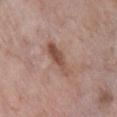Clinical impression:
Part of a total-body skin-imaging series; this lesion was reviewed on a skin check and was not flagged for biopsy.
Context:
Longest diameter approximately 4.5 mm. The lesion-visualizer software estimated an average lesion color of about L≈50 a*≈20 b*≈26 (CIELAB), a lesion–skin lightness drop of about 11, and a lesion-to-skin contrast of about 8 (normalized; higher = more distinct). The analysis additionally found a classifier nevus-likeness of about 15/100 and a detector confidence of about 100 out of 100 that the crop contains a lesion. A region of skin cropped from a whole-body photographic capture, roughly 15 mm wide. The lesion is on the right lower leg. A female subject, aged 68–72.